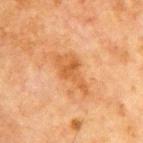location = the chest | tile lighting = cross-polarized illumination | image = total-body-photography crop, ~15 mm field of view | patient = male, aged around 65 | diameter = ~5.5 mm (longest diameter) | automated metrics = internal color variation of about 3 on a 0–10 scale and a peripheral color-asymmetry measure near 1; a classifier nevus-likeness of about 15/100.